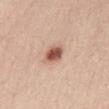Imaged during a routine full-body skin examination; the lesion was not biopsied and no histopathology is available.
From the chest.
An algorithmic analysis of the crop reported an area of roughly 5.5 mm², a shape eccentricity near 0.65, and two-axis asymmetry of about 0.15. And it measured a lesion-to-skin contrast of about 10.5 (normalized; higher = more distinct).
Imaged with white-light lighting.
The subject is a female aged approximately 25.
About 3 mm across.
A region of skin cropped from a whole-body photographic capture, roughly 15 mm wide.